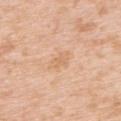workup: total-body-photography surveillance lesion; no biopsy